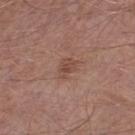  biopsy_status: not biopsied; imaged during a skin examination
  lighting: white-light
  automated_metrics:
    area_mm2_approx: 3.5
    eccentricity: 0.7
    shape_asymmetry: 0.3
    cielab_L: 47
    cielab_a: 21
    cielab_b: 26
    vs_skin_darker_L: 8.0
    vs_skin_contrast_norm: 6.0
    border_irregularity_0_10: 2.5
    peripheral_color_asymmetry: 1.0
  patient:
    sex: male
    age_approx: 55
  site: right lower leg
  image:
    source: total-body photography crop
    field_of_view_mm: 15